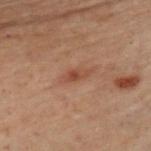{"biopsy_status": "not biopsied; imaged during a skin examination", "image": {"source": "total-body photography crop", "field_of_view_mm": 15}, "site": "chest", "patient": {"sex": "male", "age_approx": 50}, "lighting": "cross-polarized", "lesion_size": {"long_diameter_mm_approx": 3.0}, "automated_metrics": {"area_mm2_approx": 3.5, "eccentricity": 0.9}}